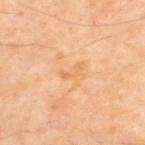Part of a total-body skin-imaging series; this lesion was reviewed on a skin check and was not flagged for biopsy.
Cropped from a total-body skin-imaging series; the visible field is about 15 mm.
The lesion is located on the back.
The lesion's longest dimension is about 2.5 mm.
A male patient approximately 65 years of age.
Imaged with cross-polarized lighting.